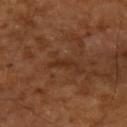Q: Was this lesion biopsied?
A: total-body-photography surveillance lesion; no biopsy
Q: What kind of image is this?
A: ~15 mm tile from a whole-body skin photo
Q: Who is the patient?
A: roughly 65 years of age
Q: Automated lesion metrics?
A: a footprint of about 3 mm², a shape eccentricity near 0.95, and two-axis asymmetry of about 0.45; about 6 CIELAB-L* units darker than the surrounding skin and a normalized border contrast of about 6; a border-irregularity rating of about 5/10, a color-variation rating of about 0/10, and a peripheral color-asymmetry measure near 0; a classifier nevus-likeness of about 0/100 and a detector confidence of about 90 out of 100 that the crop contains a lesion
Q: What is the anatomic site?
A: the arm
Q: What lighting was used for the tile?
A: cross-polarized illumination
Q: What is the lesion's diameter?
A: ~3.5 mm (longest diameter)